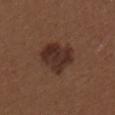Part of a total-body skin-imaging series; this lesion was reviewed on a skin check and was not flagged for biopsy. The patient is a female in their 30s. On the left upper arm. Measured at roughly 5 mm in maximum diameter. A region of skin cropped from a whole-body photographic capture, roughly 15 mm wide.